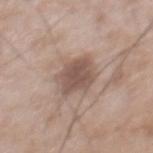workup = catalogued during a skin exam; not biopsied
lighting = white-light illumination
lesion size = ~5 mm (longest diameter)
anatomic site = the mid back
subject = male, aged 48 to 52
image = 15 mm crop, total-body photography